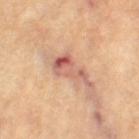Recorded during total-body skin imaging; not selected for excision or biopsy.
The lesion is located on the left thigh.
Cropped from a whole-body photographic skin survey; the tile spans about 15 mm.
A female patient aged approximately 65.